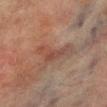<tbp_lesion>
<biopsy_status>not biopsied; imaged during a skin examination</biopsy_status>
<automated_metrics>
  <area_mm2_approx>8.0</area_mm2_approx>
  <eccentricity>0.9</eccentricity>
  <shape_asymmetry>0.65</shape_asymmetry>
</automated_metrics>
<site>right lower leg</site>
<patient>
  <sex>male</sex>
  <age_approx>70</age_approx>
</patient>
<lesion_size>
  <long_diameter_mm_approx>5.5</long_diameter_mm_approx>
</lesion_size>
<image>
  <source>total-body photography crop</source>
  <field_of_view_mm>15</field_of_view_mm>
</image>
</tbp_lesion>